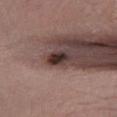– workup · no biopsy performed (imaged during a skin exam)
– automated lesion analysis · an average lesion color of about L≈30 a*≈17 b*≈18 (CIELAB), a lesion–skin lightness drop of about 17, and a normalized lesion–skin contrast near 14.5; border irregularity of about 3 on a 0–10 scale and a color-variation rating of about 3/10; an automated nevus-likeness rating near 55 out of 100 and a detector confidence of about 100 out of 100 that the crop contains a lesion
– site · the left lower leg
– patient · male, approximately 40 years of age
– tile lighting · white-light illumination
– lesion diameter · ~2.5 mm (longest diameter)
– image · total-body-photography crop, ~15 mm field of view